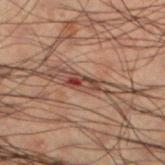Findings:
* notes — imaged on a skin check; not biopsied
* lighting — cross-polarized
* imaging modality — total-body-photography crop, ~15 mm field of view
* location — the left lower leg
* lesion size — ~3.5 mm (longest diameter)
* patient — male, approximately 50 years of age
* image-analysis metrics — a footprint of about 3.5 mm², a shape eccentricity near 0.9, and a symmetry-axis asymmetry near 0.5; a lesion color around L≈32 a*≈18 b*≈21 in CIELAB and a normalized lesion–skin contrast near 9.5; a classifier nevus-likeness of about 0/100 and a lesion-detection confidence of about 90/100